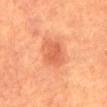Assessment:
The lesion was tiled from a total-body skin photograph and was not biopsied.
Acquisition and patient details:
From the abdomen. The recorded lesion diameter is about 3.5 mm. Cropped from a whole-body photographic skin survey; the tile spans about 15 mm. Automated image analysis of the tile measured a footprint of about 8.5 mm², a shape eccentricity near 0.7, and a shape-asymmetry score of about 0.25 (0 = symmetric). The software also gave border irregularity of about 2.5 on a 0–10 scale. The subject is a male in their mid- to late 60s. Captured under cross-polarized illumination.